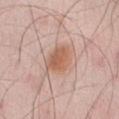follow-up — catalogued during a skin exam; not biopsied
site — the abdomen
image — 15 mm crop, total-body photography
size — ~4 mm (longest diameter)
lighting — white-light
subject — male, aged approximately 55